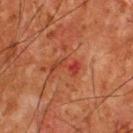workup: no biopsy performed (imaged during a skin exam)
automated lesion analysis: a lesion area of about 4.5 mm², an eccentricity of roughly 0.9, and two-axis asymmetry of about 0.45; a lesion color around L≈34 a*≈28 b*≈30 in CIELAB, a lesion–skin lightness drop of about 6, and a normalized border contrast of about 6; a border-irregularity index near 7/10 and internal color variation of about 2.5 on a 0–10 scale; a nevus-likeness score of about 0/100 and a lesion-detection confidence of about 100/100
body site: the upper back
patient: male, in their mid- to late 60s
acquisition: ~15 mm crop, total-body skin-cancer survey